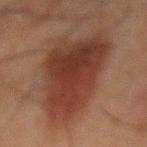The lesion was photographed on a routine skin check and not biopsied; there is no pathology result. Imaged with cross-polarized lighting. The patient is a male about 65 years old. A close-up tile cropped from a whole-body skin photograph, about 15 mm across. About 10.5 mm across. The lesion is located on the mid back.